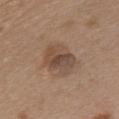Findings:
- workup: imaged on a skin check; not biopsied
- body site: the chest
- subject: male, roughly 50 years of age
- image: 15 mm crop, total-body photography
- lesion size: about 4 mm
- automated metrics: a mean CIELAB color near L≈47 a*≈16 b*≈26, about 9 CIELAB-L* units darker than the surrounding skin, and a normalized border contrast of about 7.5; a border-irregularity index near 2.5/10, a within-lesion color-variation index near 5.5/10, and peripheral color asymmetry of about 1.5